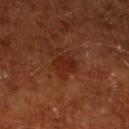Part of a total-body skin-imaging series; this lesion was reviewed on a skin check and was not flagged for biopsy. A male subject, in their mid-60s. A 15 mm close-up tile from a total-body photography series done for melanoma screening. The lesion is on the left lower leg.